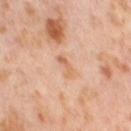Impression:
This lesion was catalogued during total-body skin photography and was not selected for biopsy.
Image and clinical context:
The lesion is on the right thigh. A female subject, about 55 years old. A roughly 15 mm field-of-view crop from a total-body skin photograph.